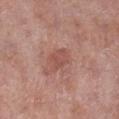| feature | finding |
|---|---|
| patient | male, aged approximately 70 |
| diameter | ~2.5 mm (longest diameter) |
| tile lighting | white-light illumination |
| automated metrics | a mean CIELAB color near L≈51 a*≈25 b*≈26 and roughly 7 lightness units darker than nearby skin; a border-irregularity index near 2/10, a color-variation rating of about 2.5/10, and peripheral color asymmetry of about 1 |
| location | the right lower leg |
| image source | total-body-photography crop, ~15 mm field of view |